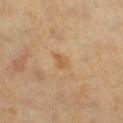No biopsy was performed on this lesion — it was imaged during a full skin examination and was not determined to be concerning. The total-body-photography lesion software estimated a lesion area of about 3.5 mm², an eccentricity of roughly 0.75, and two-axis asymmetry of about 0.25. The analysis additionally found a nevus-likeness score of about 0/100 and lesion-presence confidence of about 100/100. A lesion tile, about 15 mm wide, cut from a 3D total-body photograph. Imaged with cross-polarized lighting. The lesion is located on the right lower leg. The recorded lesion diameter is about 2.5 mm. A female subject, aged approximately 60.